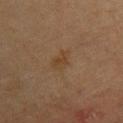A female subject, in their mid- to late 60s. Measured at roughly 3.5 mm in maximum diameter. Cropped from a whole-body photographic skin survey; the tile spans about 15 mm. Located on the upper back. Captured under cross-polarized illumination.